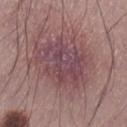follow-up = catalogued during a skin exam; not biopsied
body site = the right lower leg
illumination = white-light
image = total-body-photography crop, ~15 mm field of view
subject = male, aged 33–37